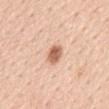Q: Is there a histopathology result?
A: catalogued during a skin exam; not biopsied